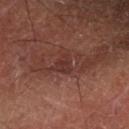Clinical impression:
Recorded during total-body skin imaging; not selected for excision or biopsy.
Clinical summary:
A lesion tile, about 15 mm wide, cut from a 3D total-body photograph. The patient is a male about 70 years old. The lesion-visualizer software estimated a mean CIELAB color near L≈23 a*≈17 b*≈17, a lesion–skin lightness drop of about 5, and a normalized border contrast of about 5.5. And it measured a border-irregularity index near 3/10, a within-lesion color-variation index near 1.5/10, and peripheral color asymmetry of about 0.5. And it measured a nevus-likeness score of about 0/100. Located on the left forearm. Measured at roughly 3 mm in maximum diameter.